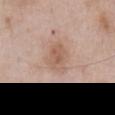biopsy_status: not biopsied; imaged during a skin examination
patient:
  sex: male
  age_approx: 60
site: front of the torso
image:
  source: total-body photography crop
  field_of_view_mm: 15
lesion_size:
  long_diameter_mm_approx: 4.0
automated_metrics:
  border_irregularity_0_10: 2.5
  color_variation_0_10: 3.0
  peripheral_color_asymmetry: 1.0
  lesion_detection_confidence_0_100: 100